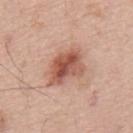Captured during whole-body skin photography for melanoma surveillance; the lesion was not biopsied. A male subject roughly 65 years of age. On the mid back. This is a white-light tile. A 15 mm close-up extracted from a 3D total-body photography capture.